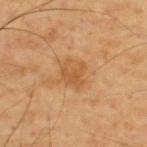Impression:
No biopsy was performed on this lesion — it was imaged during a full skin examination and was not determined to be concerning.
Background:
A region of skin cropped from a whole-body photographic capture, roughly 15 mm wide. The lesion is on the upper back. A male subject, approximately 55 years of age. The tile uses cross-polarized illumination.